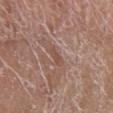No biopsy was performed on this lesion — it was imaged during a full skin examination and was not determined to be concerning.
The total-body-photography lesion software estimated a footprint of about 2 mm², an outline eccentricity of about 0.85 (0 = round, 1 = elongated), and a symmetry-axis asymmetry near 0.4. The analysis additionally found an average lesion color of about L≈49 a*≈20 b*≈26 (CIELAB), a lesion–skin lightness drop of about 7, and a lesion-to-skin contrast of about 5.5 (normalized; higher = more distinct). It also reported a nevus-likeness score of about 0/100 and a detector confidence of about 15 out of 100 that the crop contains a lesion.
The lesion's longest dimension is about 2 mm.
A female subject, aged 78–82.
A 15 mm close-up extracted from a 3D total-body photography capture.
On the right lower leg.